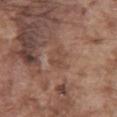<record>
  <biopsy_status>not biopsied; imaged during a skin examination</biopsy_status>
  <automated_metrics>
    <nevus_likeness_0_100>0</nevus_likeness_0_100>
    <lesion_detection_confidence_0_100>95</lesion_detection_confidence_0_100>
  </automated_metrics>
  <lighting>white-light</lighting>
  <image>
    <source>total-body photography crop</source>
    <field_of_view_mm>15</field_of_view_mm>
  </image>
  <lesion_size>
    <long_diameter_mm_approx>2.5</long_diameter_mm_approx>
  </lesion_size>
  <site>front of the torso</site>
  <patient>
    <sex>male</sex>
    <age_approx>75</age_approx>
  </patient>
</record>